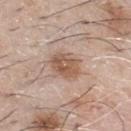This lesion was catalogued during total-body skin photography and was not selected for biopsy. About 4 mm across. The subject is a male aged approximately 65. From the front of the torso. A roughly 15 mm field-of-view crop from a total-body skin photograph.